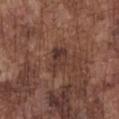Part of a total-body skin-imaging series; this lesion was reviewed on a skin check and was not flagged for biopsy.
Measured at roughly 3.5 mm in maximum diameter.
A lesion tile, about 15 mm wide, cut from a 3D total-body photograph.
A male patient, approximately 75 years of age.
Captured under white-light illumination.
Located on the front of the torso.